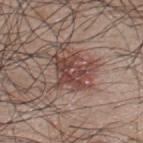Findings:
• notes · imaged on a skin check; not biopsied
• acquisition · ~15 mm crop, total-body skin-cancer survey
• tile lighting · white-light
• TBP lesion metrics · a mean CIELAB color near L≈43 a*≈20 b*≈23, a lesion–skin lightness drop of about 10, and a normalized border contrast of about 8
• lesion size · ~3.5 mm (longest diameter)
• patient · male, in their mid-40s
• location · the upper back